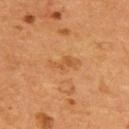Impression:
The lesion was photographed on a routine skin check and not biopsied; there is no pathology result.
Context:
The lesion is located on the back. This is a cross-polarized tile. A region of skin cropped from a whole-body photographic capture, roughly 15 mm wide. A male patient, aged 53–57.